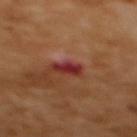Q: Is there a histopathology result?
A: no biopsy performed (imaged during a skin exam)
Q: What is the imaging modality?
A: ~15 mm crop, total-body skin-cancer survey
Q: What did automated image analysis measure?
A: a lesion area of about 3.5 mm², an outline eccentricity of about 0.8 (0 = round, 1 = elongated), and a shape-asymmetry score of about 0.25 (0 = symmetric)
Q: What lighting was used for the tile?
A: cross-polarized illumination
Q: What is the anatomic site?
A: the upper back
Q: How large is the lesion?
A: ~2.5 mm (longest diameter)
Q: What are the patient's age and sex?
A: female, aged approximately 55